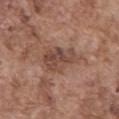The lesion was tiled from a total-body skin photograph and was not biopsied. Imaged with white-light lighting. The patient is a male in their mid- to late 70s. The lesion is on the mid back. A region of skin cropped from a whole-body photographic capture, roughly 15 mm wide. Longest diameter approximately 5.5 mm.